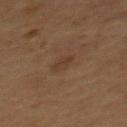notes: imaged on a skin check; not biopsied
tile lighting: cross-polarized illumination
location: the mid back
subject: male, aged approximately 55
automated lesion analysis: an automated nevus-likeness rating near 0 out of 100 and a lesion-detection confidence of about 100/100
image source: total-body-photography crop, ~15 mm field of view